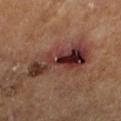<lesion>
<biopsy_status>not biopsied; imaged during a skin examination</biopsy_status>
<image>
  <source>total-body photography crop</source>
  <field_of_view_mm>15</field_of_view_mm>
</image>
<lesion_size>
  <long_diameter_mm_approx>8.0</long_diameter_mm_approx>
</lesion_size>
<patient>
  <sex>male</sex>
  <age_approx>65</age_approx>
</patient>
<site>leg</site>
<lighting>cross-polarized</lighting>
<automated_metrics>
  <area_mm2_approx>22.0</area_mm2_approx>
  <shape_asymmetry>0.35</shape_asymmetry>
  <nevus_likeness_0_100>0</nevus_likeness_0_100>
  <lesion_detection_confidence_0_100>100</lesion_detection_confidence_0_100>
</automated_metrics>
</lesion>